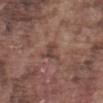biopsy_status: not biopsied; imaged during a skin examination
patient:
  sex: male
  age_approx: 75
lighting: white-light
lesion_size:
  long_diameter_mm_approx: 2.5
site: abdomen
image:
  source: total-body photography crop
  field_of_view_mm: 15
automated_metrics:
  area_mm2_approx: 3.5
  eccentricity: 0.7
  shape_asymmetry: 0.4
  cielab_L: 42
  cielab_a: 18
  cielab_b: 22
  vs_skin_darker_L: 8.0
  vs_skin_contrast_norm: 7.0
  border_irregularity_0_10: 4.5
  color_variation_0_10: 2.0
  nevus_likeness_0_100: 0
  lesion_detection_confidence_0_100: 95